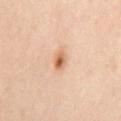The lesion was photographed on a routine skin check and not biopsied; there is no pathology result. Automated tile analysis of the lesion measured border irregularity of about 2.5 on a 0–10 scale, a within-lesion color-variation index near 7/10, and a peripheral color-asymmetry measure near 2.5. Cropped from a total-body skin-imaging series; the visible field is about 15 mm. A female subject, roughly 40 years of age. From the mid back.